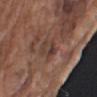| key | value |
|---|---|
| biopsy status | catalogued during a skin exam; not biopsied |